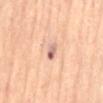{
  "biopsy_status": "not biopsied; imaged during a skin examination",
  "patient": {
    "sex": "male",
    "age_approx": 65
  },
  "image": {
    "source": "total-body photography crop",
    "field_of_view_mm": 15
  },
  "site": "abdomen"
}